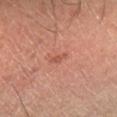biopsy_status: not biopsied; imaged during a skin examination
image:
  source: total-body photography crop
  field_of_view_mm: 15
patient:
  age_approx: 65
site: right lower leg